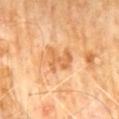Clinical impression:
Imaged during a routine full-body skin examination; the lesion was not biopsied and no histopathology is available.
Background:
On the abdomen. A roughly 15 mm field-of-view crop from a total-body skin photograph. A male patient in their 60s.